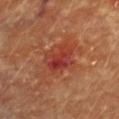Findings:
* notes — no biopsy performed (imaged during a skin exam)
* illumination — cross-polarized
* anatomic site — the chest
* subject — female, about 80 years old
* imaging modality — ~15 mm crop, total-body skin-cancer survey
* lesion diameter — ~4 mm (longest diameter)